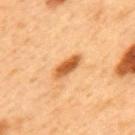From the back. Measured at roughly 4 mm in maximum diameter. A male patient aged 48 to 52. A 15 mm crop from a total-body photograph taken for skin-cancer surveillance.